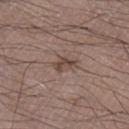Clinical impression:
Recorded during total-body skin imaging; not selected for excision or biopsy.
Context:
Approximately 3 mm at its widest. This is a white-light tile. The lesion is located on the leg. Automated tile analysis of the lesion measured a lesion area of about 3.5 mm², a shape eccentricity near 0.85, and two-axis asymmetry of about 0.3. The software also gave a mean CIELAB color near L≈44 a*≈16 b*≈22, roughly 9 lightness units darker than nearby skin, and a normalized border contrast of about 7.5. The software also gave a border-irregularity index near 3/10. The software also gave a detector confidence of about 100 out of 100 that the crop contains a lesion. A 15 mm close-up tile from a total-body photography series done for melanoma screening. A male patient in their mid- to late 70s.